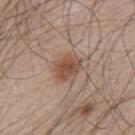The lesion was tiled from a total-body skin photograph and was not biopsied. Located on the upper back. The patient is a male aged 48 to 52. The recorded lesion diameter is about 3.5 mm. A 15 mm crop from a total-body photograph taken for skin-cancer surveillance.